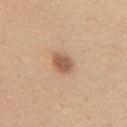Clinical impression: Recorded during total-body skin imaging; not selected for excision or biopsy. Image and clinical context: Located on the mid back. A 15 mm crop from a total-body photograph taken for skin-cancer surveillance. A female subject aged 43 to 47. The lesion-visualizer software estimated a border-irregularity index near 1.5/10 and a peripheral color-asymmetry measure near 1. It also reported an automated nevus-likeness rating near 95 out of 100. Approximately 3 mm at its widest.